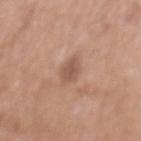The lesion is on the mid back.
A male patient, approximately 70 years of age.
About 3 mm across.
Cropped from a total-body skin-imaging series; the visible field is about 15 mm.